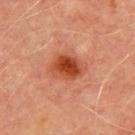The lesion's longest dimension is about 4 mm. This is a cross-polarized tile. On the chest. A region of skin cropped from a whole-body photographic capture, roughly 15 mm wide. A male subject aged approximately 70.